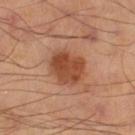Imaged during a routine full-body skin examination; the lesion was not biopsied and no histopathology is available.
The recorded lesion diameter is about 4.5 mm.
Located on the right thigh.
Automated tile analysis of the lesion measured a footprint of about 13 mm², an eccentricity of roughly 0.6, and a symmetry-axis asymmetry near 0.2. The analysis additionally found an average lesion color of about L≈48 a*≈26 b*≈35 (CIELAB) and about 12 CIELAB-L* units darker than the surrounding skin.
Captured under cross-polarized illumination.
A roughly 15 mm field-of-view crop from a total-body skin photograph.